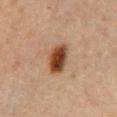Clinical impression:
The lesion was photographed on a routine skin check and not biopsied; there is no pathology result.
Image and clinical context:
A male patient aged 63 to 67. The total-body-photography lesion software estimated a mean CIELAB color near L≈38 a*≈19 b*≈29, about 15 CIELAB-L* units darker than the surrounding skin, and a lesion-to-skin contrast of about 12.5 (normalized; higher = more distinct). The analysis additionally found a border-irregularity rating of about 2/10 and radial color variation of about 1.5. The software also gave a nevus-likeness score of about 100/100 and lesion-presence confidence of about 100/100. Imaged with cross-polarized lighting. Cropped from a total-body skin-imaging series; the visible field is about 15 mm. From the abdomen. About 4 mm across.